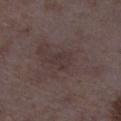A female subject aged around 35. On the left lower leg. This image is a 15 mm lesion crop taken from a total-body photograph.